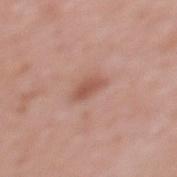image-analysis metrics=a lesion area of about 4.5 mm², a shape eccentricity near 0.85, and a symmetry-axis asymmetry near 0.25 | anatomic site=the mid back | subject=female, aged approximately 40 | acquisition=~15 mm crop, total-body skin-cancer survey | size=≈3 mm | tile lighting=white-light.